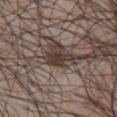The lesion was tiled from a total-body skin photograph and was not biopsied. Captured under white-light illumination. The patient is a male about 70 years old. A 15 mm close-up tile from a total-body photography series done for melanoma screening. Longest diameter approximately 3.5 mm. The lesion-visualizer software estimated a border-irregularity rating of about 4/10 and peripheral color asymmetry of about 1. The lesion is on the chest.